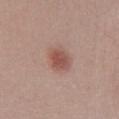Assessment: No biopsy was performed on this lesion — it was imaged during a full skin examination and was not determined to be concerning.